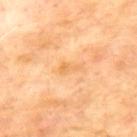<record>
<biopsy_status>not biopsied; imaged during a skin examination</biopsy_status>
<lighting>cross-polarized</lighting>
<image>
  <source>total-body photography crop</source>
  <field_of_view_mm>15</field_of_view_mm>
</image>
<site>upper back</site>
<patient>
  <sex>male</sex>
  <age_approx>65</age_approx>
</patient>
<automated_metrics>
  <area_mm2_approx>2.5</area_mm2_approx>
  <eccentricity>0.9</eccentricity>
  <shape_asymmetry>0.6</shape_asymmetry>
  <border_irregularity_0_10>6.0</border_irregularity_0_10>
  <color_variation_0_10>0.0</color_variation_0_10>
  <lesion_detection_confidence_0_100>100</lesion_detection_confidence_0_100>
</automated_metrics>
</record>